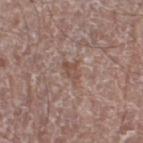The lesion was photographed on a routine skin check and not biopsied; there is no pathology result. A 15 mm close-up tile from a total-body photography series done for melanoma screening. The subject is a male aged 73 to 77. About 2.5 mm across. Captured under white-light illumination. Automated tile analysis of the lesion measured a lesion area of about 3.5 mm², an eccentricity of roughly 0.8, and a shape-asymmetry score of about 0.45 (0 = symmetric). And it measured an average lesion color of about L≈49 a*≈18 b*≈24 (CIELAB), about 8 CIELAB-L* units darker than the surrounding skin, and a lesion-to-skin contrast of about 6 (normalized; higher = more distinct). It also reported a border-irregularity index near 4.5/10 and peripheral color asymmetry of about 0.5. It also reported an automated nevus-likeness rating near 0 out of 100. Located on the left lower leg.